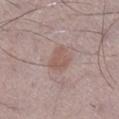<tbp_lesion>
  <biopsy_status>not biopsied; imaged during a skin examination</biopsy_status>
  <patient>
    <sex>male</sex>
    <age_approx>50</age_approx>
  </patient>
  <automated_metrics>
    <area_mm2_approx>6.0</area_mm2_approx>
    <shape_asymmetry>0.3</shape_asymmetry>
    <border_irregularity_0_10>2.5</border_irregularity_0_10>
    <peripheral_color_asymmetry>0.5</peripheral_color_asymmetry>
    <nevus_likeness_0_100>60</nevus_likeness_0_100>
    <lesion_detection_confidence_0_100>100</lesion_detection_confidence_0_100>
  </automated_metrics>
  <lighting>white-light</lighting>
  <image>
    <source>total-body photography crop</source>
    <field_of_view_mm>15</field_of_view_mm>
  </image>
  <site>right lower leg</site>
</tbp_lesion>